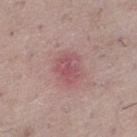No biopsy was performed on this lesion — it was imaged during a full skin examination and was not determined to be concerning.